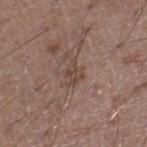Assessment:
Imaged during a routine full-body skin examination; the lesion was not biopsied and no histopathology is available.
Context:
A male subject in their 20s. A close-up tile cropped from a whole-body skin photograph, about 15 mm across. On the right lower leg. An algorithmic analysis of the crop reported an area of roughly 3.5 mm² and an outline eccentricity of about 0.75 (0 = round, 1 = elongated). It also reported a mean CIELAB color near L≈44 a*≈17 b*≈23, a lesion–skin lightness drop of about 7, and a lesion-to-skin contrast of about 6 (normalized; higher = more distinct). The analysis additionally found a border-irregularity rating of about 4/10, a within-lesion color-variation index near 2.5/10, and a peripheral color-asymmetry measure near 0.5. This is a white-light tile. The lesion's longest dimension is about 3 mm.